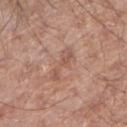notes: no biopsy performed (imaged during a skin exam) | size: ≈4.5 mm | TBP lesion metrics: an area of roughly 5.5 mm², an outline eccentricity of about 0.95 (0 = round, 1 = elongated), and a shape-asymmetry score of about 0.5 (0 = symmetric); a nevus-likeness score of about 0/100 and a lesion-detection confidence of about 100/100 | acquisition: total-body-photography crop, ~15 mm field of view | location: the right forearm | subject: male, aged 63–67 | tile lighting: white-light illumination.